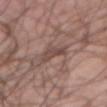Q: Was this lesion biopsied?
A: total-body-photography surveillance lesion; no biopsy
Q: What kind of image is this?
A: total-body-photography crop, ~15 mm field of view
Q: Illumination type?
A: white-light illumination
Q: How large is the lesion?
A: ~2.5 mm (longest diameter)
Q: Lesion location?
A: the abdomen
Q: Who is the patient?
A: male, about 55 years old